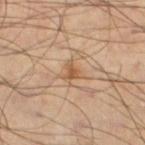{
  "biopsy_status": "not biopsied; imaged during a skin examination",
  "lesion_size": {
    "long_diameter_mm_approx": 2.5
  },
  "patient": {
    "sex": "male",
    "age_approx": 45
  },
  "image": {
    "source": "total-body photography crop",
    "field_of_view_mm": 15
  },
  "site": "left lower leg",
  "automated_metrics": {
    "eccentricity": 0.5,
    "shape_asymmetry": 0.5,
    "cielab_L": 54,
    "cielab_a": 18,
    "cielab_b": 34,
    "vs_skin_darker_L": 9.0,
    "vs_skin_contrast_norm": 7.0,
    "nevus_likeness_0_100": 0,
    "lesion_detection_confidence_0_100": 100
  },
  "lighting": "cross-polarized"
}